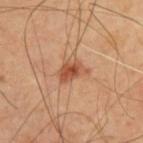illumination — cross-polarized illumination | anatomic site — the upper back | TBP lesion metrics — an outline eccentricity of about 0.75 (0 = round, 1 = elongated) and a symmetry-axis asymmetry near 0.35; a nevus-likeness score of about 90/100 and a detector confidence of about 100 out of 100 that the crop contains a lesion | lesion diameter — ~3.5 mm (longest diameter) | image source — ~15 mm tile from a whole-body skin photo | patient — male, roughly 65 years of age.